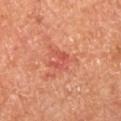<case>
<biopsy_status>not biopsied; imaged during a skin examination</biopsy_status>
<site>right lower leg</site>
<patient>
  <sex>male</sex>
  <age_approx>65</age_approx>
</patient>
<lighting>cross-polarized</lighting>
<image>
  <source>total-body photography crop</source>
  <field_of_view_mm>15</field_of_view_mm>
</image>
</case>